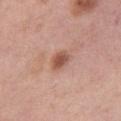Captured during whole-body skin photography for melanoma surveillance; the lesion was not biopsied.
A lesion tile, about 15 mm wide, cut from a 3D total-body photograph.
Longest diameter approximately 2.5 mm.
The lesion-visualizer software estimated a footprint of about 4.5 mm² and a shape eccentricity near 0.65. And it measured an automated nevus-likeness rating near 90 out of 100 and a lesion-detection confidence of about 100/100.
A female patient, aged 48–52.
The lesion is located on the leg.
Imaged with white-light lighting.